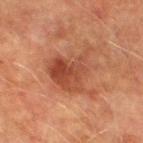Findings:
– anatomic site — the left thigh
– patient — male, in their mid-70s
– image — ~15 mm crop, total-body skin-cancer survey